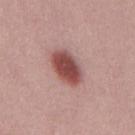Notes:
• subject · male, aged around 30
• image · 15 mm crop, total-body photography